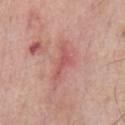Image and clinical context: The total-body-photography lesion software estimated a mean CIELAB color near L≈55 a*≈30 b*≈24 and about 9 CIELAB-L* units darker than the surrounding skin. The software also gave a border-irregularity index near 6.5/10, internal color variation of about 1 on a 0–10 scale, and peripheral color asymmetry of about 0.5. And it measured a classifier nevus-likeness of about 0/100 and a detector confidence of about 85 out of 100 that the crop contains a lesion. The tile uses white-light illumination. A male subject about 60 years old. A 15 mm close-up extracted from a 3D total-body photography capture. Approximately 4 mm at its widest. On the chest.